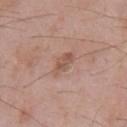No biopsy was performed on this lesion — it was imaged during a full skin examination and was not determined to be concerning.
From the chest.
A roughly 15 mm field-of-view crop from a total-body skin photograph.
A male subject about 65 years old.